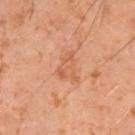Q: How was this image acquired?
A: total-body-photography crop, ~15 mm field of view
Q: Who is the patient?
A: male, aged 48–52
Q: How large is the lesion?
A: ~3.5 mm (longest diameter)
Q: Lesion location?
A: the arm
Q: How was the tile lit?
A: cross-polarized illumination